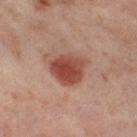<lesion>
  <biopsy_status>not biopsied; imaged during a skin examination</biopsy_status>
  <lesion_size>
    <long_diameter_mm_approx>4.5</long_diameter_mm_approx>
  </lesion_size>
  <site>left thigh</site>
  <automated_metrics>
    <area_mm2_approx>12.0</area_mm2_approx>
    <shape_asymmetry>0.25</shape_asymmetry>
  </automated_metrics>
  <lighting>cross-polarized</lighting>
  <patient>
    <sex>female</sex>
    <age_approx>55</age_approx>
  </patient>
  <image>
    <source>total-body photography crop</source>
    <field_of_view_mm>15</field_of_view_mm>
  </image>
</lesion>